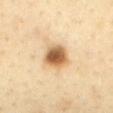The lesion was photographed on a routine skin check and not biopsied; there is no pathology result. The patient is a female aged 38 to 42. The lesion's longest dimension is about 3.5 mm. This is a cross-polarized tile. A 15 mm crop from a total-body photograph taken for skin-cancer surveillance. The lesion is on the mid back.